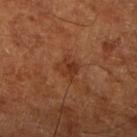Case summary:
• biopsy status — imaged on a skin check; not biopsied
• illumination — cross-polarized illumination
• location — the right lower leg
• patient — aged 63 to 67
• image source — ~15 mm tile from a whole-body skin photo